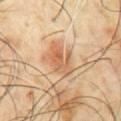notes: imaged on a skin check; not biopsied
size: ~4.5 mm (longest diameter)
TBP lesion metrics: an eccentricity of roughly 0.8 and a shape-asymmetry score of about 0.3 (0 = symmetric); a lesion color around L≈60 a*≈21 b*≈36 in CIELAB and about 10 CIELAB-L* units darker than the surrounding skin; a border-irregularity index near 4.5/10, internal color variation of about 6 on a 0–10 scale, and a peripheral color-asymmetry measure near 2
image: total-body-photography crop, ~15 mm field of view
subject: male, roughly 60 years of age
anatomic site: the chest